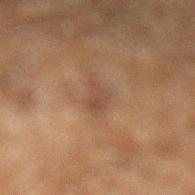Impression:
The lesion was photographed on a routine skin check and not biopsied; there is no pathology result.
Clinical summary:
Measured at roughly 3 mm in maximum diameter. A male patient aged 58 to 62. A 15 mm crop from a total-body photograph taken for skin-cancer surveillance. The lesion is located on the left lower leg.